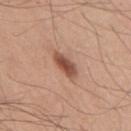Part of a total-body skin-imaging series; this lesion was reviewed on a skin check and was not flagged for biopsy. The patient is a male aged 53 to 57. The lesion-visualizer software estimated an automated nevus-likeness rating near 95 out of 100 and lesion-presence confidence of about 100/100. The lesion's longest dimension is about 4 mm. Cropped from a total-body skin-imaging series; the visible field is about 15 mm. Located on the chest.